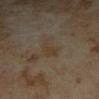Assessment:
The lesion was photographed on a routine skin check and not biopsied; there is no pathology result.
Acquisition and patient details:
From the left forearm. Imaged with cross-polarized lighting. A roughly 15 mm field-of-view crop from a total-body skin photograph. Approximately 3.5 mm at its widest. The lesion-visualizer software estimated a symmetry-axis asymmetry near 0.3. The software also gave a color-variation rating of about 0.5/10. The analysis additionally found lesion-presence confidence of about 85/100. A female subject approximately 35 years of age.